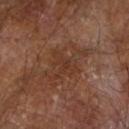Imaged during a routine full-body skin examination; the lesion was not biopsied and no histopathology is available. The subject is a male aged around 65. A lesion tile, about 15 mm wide, cut from a 3D total-body photograph. From the right forearm. The tile uses cross-polarized illumination.